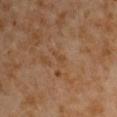Case summary:
* follow-up: no biopsy performed (imaged during a skin exam)
* body site: the left upper arm
* image source: 15 mm crop, total-body photography
* subject: male, approximately 60 years of age